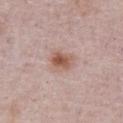Q: Was a biopsy performed?
A: no biopsy performed (imaged during a skin exam)
Q: What is the anatomic site?
A: the abdomen
Q: What are the patient's age and sex?
A: male, aged 73 to 77
Q: What did automated image analysis measure?
A: a lesion area of about 6.5 mm², a shape eccentricity near 0.7, and a shape-asymmetry score of about 0.2 (0 = symmetric); border irregularity of about 2 on a 0–10 scale, a within-lesion color-variation index near 6/10, and peripheral color asymmetry of about 2
Q: What kind of image is this?
A: 15 mm crop, total-body photography
Q: Lesion size?
A: about 3 mm
Q: Illumination type?
A: white-light illumination